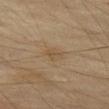  biopsy_status: not biopsied; imaged during a skin examination
  image:
    source: total-body photography crop
    field_of_view_mm: 15
  site: leg
  lighting: cross-polarized
  patient:
    sex: male
    age_approx: 70
  lesion_size:
    long_diameter_mm_approx: 2.5